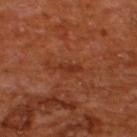Assessment:
Recorded during total-body skin imaging; not selected for excision or biopsy.
Image and clinical context:
A male patient roughly 65 years of age. This image is a 15 mm lesion crop taken from a total-body photograph. The recorded lesion diameter is about 3 mm. Imaged with cross-polarized lighting. Located on the upper back.